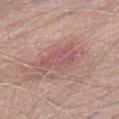This lesion was catalogued during total-body skin photography and was not selected for biopsy.
About 5.5 mm across.
The lesion-visualizer software estimated an area of roughly 11 mm², an eccentricity of roughly 0.9, and two-axis asymmetry of about 0.35. It also reported a mean CIELAB color near L≈55 a*≈23 b*≈22, about 8 CIELAB-L* units darker than the surrounding skin, and a normalized lesion–skin contrast near 5.5. And it measured lesion-presence confidence of about 85/100.
The lesion is located on the abdomen.
The patient is a male approximately 75 years of age.
This is a white-light tile.
A roughly 15 mm field-of-view crop from a total-body skin photograph.